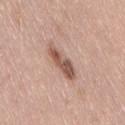<record>
  <biopsy_status>not biopsied; imaged during a skin examination</biopsy_status>
  <patient>
    <sex>female</sex>
    <age_approx>40</age_approx>
  </patient>
  <site>lower back</site>
  <image>
    <source>total-body photography crop</source>
    <field_of_view_mm>15</field_of_view_mm>
  </image>
  <lighting>white-light</lighting>
  <lesion_size>
    <long_diameter_mm_approx>5.0</long_diameter_mm_approx>
  </lesion_size>
</record>